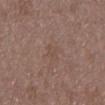Captured during whole-body skin photography for melanoma surveillance; the lesion was not biopsied. The total-body-photography lesion software estimated an area of roughly 2 mm², a shape eccentricity near 0.9, and two-axis asymmetry of about 0.4. This is a white-light tile. A 15 mm crop from a total-body photograph taken for skin-cancer surveillance. The lesion is on the mid back. The subject is a male about 50 years old.